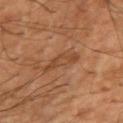The lesion was tiled from a total-body skin photograph and was not biopsied.
A close-up tile cropped from a whole-body skin photograph, about 15 mm across.
Located on the arm.
A male subject aged 48 to 52.